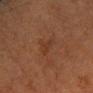workup: imaged on a skin check; not biopsied | subject: male, in their 50s | imaging modality: ~15 mm tile from a whole-body skin photo | anatomic site: the arm.